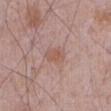Clinical impression:
The lesion was photographed on a routine skin check and not biopsied; there is no pathology result.
Background:
This is a white-light tile. A 15 mm close-up extracted from a 3D total-body photography capture. The patient is a male roughly 70 years of age. The lesion is on the abdomen.